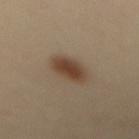Recorded during total-body skin imaging; not selected for excision or biopsy. The lesion is located on the mid back. Cropped from a total-body skin-imaging series; the visible field is about 15 mm. The subject is a female approximately 40 years of age. The tile uses cross-polarized illumination. About 4.5 mm across.